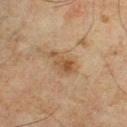- workup · catalogued during a skin exam; not biopsied
- image · ~15 mm tile from a whole-body skin photo
- tile lighting · cross-polarized
- subject · male, about 45 years old
- anatomic site · the left lower leg
- automated metrics · a lesion color around L≈42 a*≈15 b*≈29 in CIELAB and a normalized border contrast of about 6.5; a color-variation rating of about 4.5/10 and peripheral color asymmetry of about 1.5; a nevus-likeness score of about 25/100 and lesion-presence confidence of about 100/100
- lesion diameter · ~3.5 mm (longest diameter)